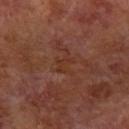Q: Was a biopsy performed?
A: no biopsy performed (imaged during a skin exam)
Q: How was this image acquired?
A: total-body-photography crop, ~15 mm field of view
Q: What did automated image analysis measure?
A: border irregularity of about 5.5 on a 0–10 scale, a color-variation rating of about 0/10, and a peripheral color-asymmetry measure near 0
Q: What are the patient's age and sex?
A: male, about 60 years old
Q: What is the anatomic site?
A: the arm